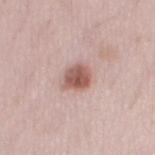Case summary:
• workup — imaged on a skin check; not biopsied
• image — 15 mm crop, total-body photography
• automated lesion analysis — roughly 14 lightness units darker than nearby skin
• patient — female, in their 30s
• illumination — white-light illumination
• body site — the left thigh